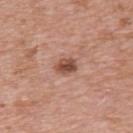{
  "biopsy_status": "not biopsied; imaged during a skin examination",
  "patient": {
    "sex": "female",
    "age_approx": 40
  },
  "lesion_size": {
    "long_diameter_mm_approx": 2.5
  },
  "site": "upper back",
  "lighting": "white-light",
  "image": {
    "source": "total-body photography crop",
    "field_of_view_mm": 15
  }
}